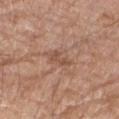Impression: Part of a total-body skin-imaging series; this lesion was reviewed on a skin check and was not flagged for biopsy. Context: This image is a 15 mm lesion crop taken from a total-body photograph. The lesion is on the right upper arm. The subject is a male aged approximately 80.